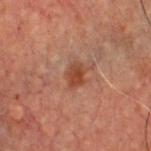No biopsy was performed on this lesion — it was imaged during a full skin examination and was not determined to be concerning. Imaged with cross-polarized lighting. A 15 mm close-up extracted from a 3D total-body photography capture. About 3 mm across. On the chest. The patient is a male approximately 60 years of age.